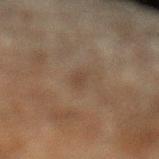<lesion>
  <biopsy_status>not biopsied; imaged during a skin examination</biopsy_status>
  <lighting>cross-polarized</lighting>
  <patient>
    <sex>male</sex>
    <age_approx>60</age_approx>
  </patient>
  <image>
    <source>total-body photography crop</source>
    <field_of_view_mm>15</field_of_view_mm>
  </image>
  <automated_metrics>
    <nevus_likeness_0_100>0</nevus_likeness_0_100>
    <lesion_detection_confidence_0_100>100</lesion_detection_confidence_0_100>
  </automated_metrics>
  <lesion_size>
    <long_diameter_mm_approx>2.5</long_diameter_mm_approx>
  </lesion_size>
  <site>left lower leg</site>
</lesion>